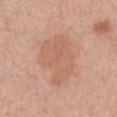illumination: white-light; image: ~15 mm tile from a whole-body skin photo; patient: female, roughly 40 years of age; location: the chest.